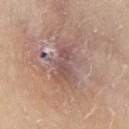Impression:
The lesion was photographed on a routine skin check and not biopsied; there is no pathology result.
Context:
Approximately 6 mm at its widest. A roughly 15 mm field-of-view crop from a total-body skin photograph. Imaged with white-light lighting. From the front of the torso. A male subject about 30 years old. Automated image analysis of the tile measured an area of roughly 16 mm² and two-axis asymmetry of about 0.35. It also reported an average lesion color of about L≈54 a*≈18 b*≈21 (CIELAB), a lesion–skin lightness drop of about 8, and a normalized lesion–skin contrast near 6.5. The software also gave a nevus-likeness score of about 0/100 and lesion-presence confidence of about 85/100.